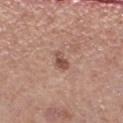{
  "biopsy_status": "not biopsied; imaged during a skin examination",
  "lighting": "white-light",
  "image": {
    "source": "total-body photography crop",
    "field_of_view_mm": 15
  },
  "site": "left lower leg",
  "patient": {
    "sex": "male",
    "age_approx": 50
  },
  "automated_metrics": {
    "area_mm2_approx": 3.5,
    "eccentricity": 0.75,
    "shape_asymmetry": 0.35,
    "cielab_L": 51,
    "cielab_a": 20,
    "cielab_b": 26,
    "vs_skin_darker_L": 11.0,
    "vs_skin_contrast_norm": 7.5,
    "border_irregularity_0_10": 3.0,
    "color_variation_0_10": 2.0,
    "peripheral_color_asymmetry": 0.5
  }
}